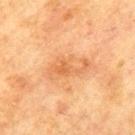Assessment:
This lesion was catalogued during total-body skin photography and was not selected for biopsy.